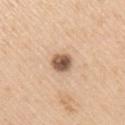  biopsy_status: not biopsied; imaged during a skin examination
  automated_metrics:
    eccentricity: 0.5
    shape_asymmetry: 0.15
    cielab_L: 57
    cielab_a: 18
    cielab_b: 31
    vs_skin_darker_L: 18.0
    vs_skin_contrast_norm: 11.0
  patient:
    sex: male
    age_approx: 60
  image:
    source: total-body photography crop
    field_of_view_mm: 15
  lighting: white-light
  lesion_size:
    long_diameter_mm_approx: 3.0
  site: right upper arm